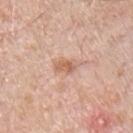Q: Is there a histopathology result?
A: no biopsy performed (imaged during a skin exam)
Q: How large is the lesion?
A: ≈3 mm
Q: Who is the patient?
A: male, aged 68–72
Q: Where on the body is the lesion?
A: the chest
Q: How was the tile lit?
A: white-light illumination
Q: How was this image acquired?
A: total-body-photography crop, ~15 mm field of view